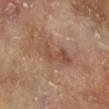This lesion was catalogued during total-body skin photography and was not selected for biopsy. A male patient, roughly 65 years of age. Automated image analysis of the tile measured an average lesion color of about L≈49 a*≈20 b*≈29 (CIELAB), roughly 8 lightness units darker than nearby skin, and a lesion-to-skin contrast of about 6 (normalized; higher = more distinct). Captured under cross-polarized illumination. A roughly 15 mm field-of-view crop from a total-body skin photograph. The lesion is on the right lower leg.